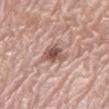No biopsy was performed on this lesion — it was imaged during a full skin examination and was not determined to be concerning. On the right lower leg. The recorded lesion diameter is about 3.5 mm. A female patient, roughly 60 years of age. Captured under white-light illumination. The lesion-visualizer software estimated an area of roughly 7 mm² and a symmetry-axis asymmetry near 0.35. And it measured an average lesion color of about L≈54 a*≈20 b*≈24 (CIELAB), roughly 13 lightness units darker than nearby skin, and a normalized border contrast of about 8.5. And it measured a border-irregularity index near 3.5/10, a color-variation rating of about 5/10, and peripheral color asymmetry of about 1. Cropped from a total-body skin-imaging series; the visible field is about 15 mm.